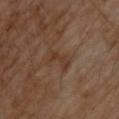workup: imaged on a skin check; not biopsied
imaging modality: ~15 mm tile from a whole-body skin photo
lesion size: ≈3.5 mm
site: the upper back
tile lighting: cross-polarized
subject: male, in their 70s